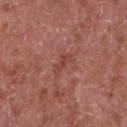| key | value |
|---|---|
| follow-up | no biopsy performed (imaged during a skin exam) |
| image | ~15 mm tile from a whole-body skin photo |
| lighting | white-light |
| patient | male, in their mid- to late 60s |
| location | the chest |